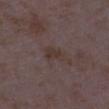Q: Was a biopsy performed?
A: total-body-photography surveillance lesion; no biopsy
Q: What did automated image analysis measure?
A: an area of roughly 4.5 mm², a shape eccentricity near 0.75, and two-axis asymmetry of about 0.3; a mean CIELAB color near L≈34 a*≈13 b*≈18, a lesion–skin lightness drop of about 5, and a normalized lesion–skin contrast near 6.5; a border-irregularity rating of about 3/10, a within-lesion color-variation index near 2/10, and peripheral color asymmetry of about 0.5
Q: Who is the patient?
A: female, aged 33–37
Q: How was this image acquired?
A: ~15 mm tile from a whole-body skin photo
Q: Lesion size?
A: ~3 mm (longest diameter)
Q: Where on the body is the lesion?
A: the right lower leg